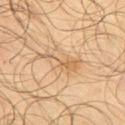Clinical impression:
The lesion was photographed on a routine skin check and not biopsied; there is no pathology result.
Acquisition and patient details:
A region of skin cropped from a whole-body photographic capture, roughly 15 mm wide. The lesion-visualizer software estimated an average lesion color of about L≈60 a*≈17 b*≈37 (CIELAB) and roughly 8 lightness units darker than nearby skin. The lesion is located on the right upper arm. About 5 mm across. This is a cross-polarized tile. A male patient approximately 65 years of age.